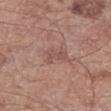Impression: Captured during whole-body skin photography for melanoma surveillance; the lesion was not biopsied. Context: Located on the left thigh. Measured at roughly 2.5 mm in maximum diameter. A 15 mm close-up extracted from a 3D total-body photography capture. Captured under white-light illumination. The total-body-photography lesion software estimated an average lesion color of about L≈51 a*≈21 b*≈23 (CIELAB) and a normalized border contrast of about 5. And it measured a nevus-likeness score of about 0/100 and a lesion-detection confidence of about 100/100. A male subject aged around 55.